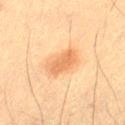Q: Was a biopsy performed?
A: imaged on a skin check; not biopsied
Q: How was this image acquired?
A: 15 mm crop, total-body photography
Q: Patient demographics?
A: male, roughly 50 years of age
Q: Lesion location?
A: the left thigh
Q: What is the lesion's diameter?
A: ≈3.5 mm
Q: What lighting was used for the tile?
A: cross-polarized